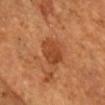{"biopsy_status": "not biopsied; imaged during a skin examination", "image": {"source": "total-body photography crop", "field_of_view_mm": 15}, "site": "head or neck", "patient": {"sex": "male", "age_approx": 55}}